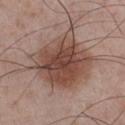Part of a total-body skin-imaging series; this lesion was reviewed on a skin check and was not flagged for biopsy.